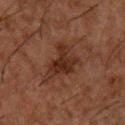workup = imaged on a skin check; not biopsied
patient = male, aged 48–52
illumination = cross-polarized illumination
acquisition = 15 mm crop, total-body photography
lesion diameter = ≈5.5 mm
location = the chest